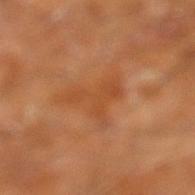biopsy_status: not biopsied; imaged during a skin examination
automated_metrics:
  eccentricity: 0.65
  shape_asymmetry: 0.8
  cielab_L: 46
  cielab_a: 26
  cielab_b: 39
  vs_skin_darker_L: 6.0
  vs_skin_contrast_norm: 5.0
lighting: cross-polarized
lesion_size:
  long_diameter_mm_approx: 4.0
site: right leg
image:
  source: total-body photography crop
  field_of_view_mm: 15
patient:
  sex: male
  age_approx: 60